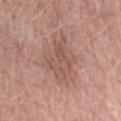The lesion was photographed on a routine skin check and not biopsied; there is no pathology result. Cropped from a whole-body photographic skin survey; the tile spans about 15 mm. Automated image analysis of the tile measured an area of roughly 16 mm², an eccentricity of roughly 0.6, and two-axis asymmetry of about 0.3. From the left forearm. The subject is a female in their 70s. About 5 mm across.